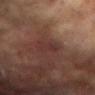This lesion was catalogued during total-body skin photography and was not selected for biopsy. Located on the right arm. A 15 mm close-up tile from a total-body photography series done for melanoma screening. A female patient, approximately 80 years of age.